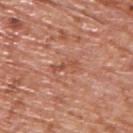biopsy_status: not biopsied; imaged during a skin examination
automated_metrics:
  area_mm2_approx: 3.0
  eccentricity: 0.95
  shape_asymmetry: 0.45
  cielab_L: 52
  cielab_a: 26
  cielab_b: 33
  vs_skin_darker_L: 8.0
  nevus_likeness_0_100: 0
patient:
  sex: male
  age_approx: 65
lighting: white-light
site: upper back
image:
  source: total-body photography crop
  field_of_view_mm: 15
lesion_size:
  long_diameter_mm_approx: 3.0